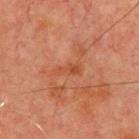biopsy status=catalogued during a skin exam; not biopsied
image source=total-body-photography crop, ~15 mm field of view
lesion size=~3.5 mm (longest diameter)
tile lighting=cross-polarized
patient=male, about 70 years old
location=the front of the torso